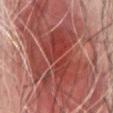  biopsy_status: not biopsied; imaged during a skin examination
  patient:
    sex: male
    age_approx: 65
  site: chest
  automated_metrics:
    area_mm2_approx: 60.0
    eccentricity: 0.9
    shape_asymmetry: 0.55
    nevus_likeness_0_100: 25
    lesion_detection_confidence_0_100: 65
  lighting: cross-polarized
  lesion_size:
    long_diameter_mm_approx: 16.0
  image:
    source: total-body photography crop
    field_of_view_mm: 15